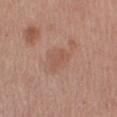Part of a total-body skin-imaging series; this lesion was reviewed on a skin check and was not flagged for biopsy.